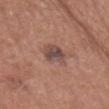No biopsy was performed on this lesion — it was imaged during a full skin examination and was not determined to be concerning.
From the head or neck.
This image is a 15 mm lesion crop taken from a total-body photograph.
This is a white-light tile.
The recorded lesion diameter is about 3.5 mm.
A female patient, aged around 80.